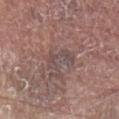Captured during whole-body skin photography for melanoma surveillance; the lesion was not biopsied.
The tile uses white-light illumination.
The subject is a male aged 78–82.
Cropped from a whole-body photographic skin survey; the tile spans about 15 mm.
Automated image analysis of the tile measured an area of roughly 5.5 mm², an eccentricity of roughly 0.85, and two-axis asymmetry of about 0.45. It also reported an average lesion color of about L≈47 a*≈15 b*≈18 (CIELAB), roughly 6 lightness units darker than nearby skin, and a lesion-to-skin contrast of about 5.5 (normalized; higher = more distinct).
About 4 mm across.
Located on the abdomen.